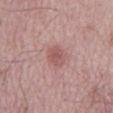Findings:
* site: the mid back
* imaging modality: ~15 mm crop, total-body skin-cancer survey
* lesion diameter: ≈3 mm
* lighting: white-light illumination
* patient: male, aged 63–67